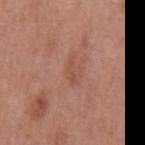Assessment:
This lesion was catalogued during total-body skin photography and was not selected for biopsy.
Context:
The lesion is located on the mid back. This is a white-light tile. The subject is a male aged 68 to 72. A lesion tile, about 15 mm wide, cut from a 3D total-body photograph.